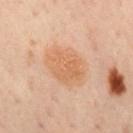Clinical impression: Captured during whole-body skin photography for melanoma surveillance; the lesion was not biopsied. Context: The lesion is on the mid back. Cropped from a whole-body photographic skin survey; the tile spans about 15 mm. A male patient in their 50s. The recorded lesion diameter is about 5.5 mm. This is a cross-polarized tile. Automated tile analysis of the lesion measured an area of roughly 16 mm², a shape eccentricity near 0.75, and two-axis asymmetry of about 0.15. And it measured a lesion color around L≈52 a*≈18 b*≈31 in CIELAB, roughly 6 lightness units darker than nearby skin, and a lesion-to-skin contrast of about 6 (normalized; higher = more distinct). It also reported a border-irregularity index near 1.5/10, a color-variation rating of about 2.5/10, and a peripheral color-asymmetry measure near 1. The software also gave an automated nevus-likeness rating near 60 out of 100.